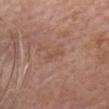Notes:
– diameter: about 2.5 mm
– location: the head or neck
– automated lesion analysis: an area of roughly 2.5 mm²; a mean CIELAB color near L≈51 a*≈20 b*≈30, roughly 5 lightness units darker than nearby skin, and a normalized lesion–skin contrast near 4.5; a border-irregularity rating of about 3.5/10 and a peripheral color-asymmetry measure near 0
– subject: female, roughly 70 years of age
– imaging modality: total-body-photography crop, ~15 mm field of view
– tile lighting: white-light